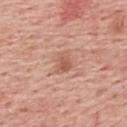Clinical impression: The lesion was photographed on a routine skin check and not biopsied; there is no pathology result. Background: The total-body-photography lesion software estimated an area of roughly 4.5 mm². It also reported a mean CIELAB color near L≈58 a*≈23 b*≈30, about 9 CIELAB-L* units darker than the surrounding skin, and a normalized border contrast of about 6. The software also gave a border-irregularity rating of about 2/10 and a within-lesion color-variation index near 3/10. The analysis additionally found lesion-presence confidence of about 100/100. A 15 mm close-up tile from a total-body photography series done for melanoma screening. The lesion's longest dimension is about 2.5 mm. A male subject aged approximately 60. Located on the upper back. This is a white-light tile.